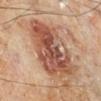Clinical impression:
Recorded during total-body skin imaging; not selected for excision or biopsy.
Acquisition and patient details:
A male subject, in their 70s. Located on the right lower leg. Cropped from a total-body skin-imaging series; the visible field is about 15 mm. Measured at roughly 8 mm in maximum diameter. Automated image analysis of the tile measured a lesion area of about 30 mm², an eccentricity of roughly 0.75, and a shape-asymmetry score of about 0.3 (0 = symmetric). The analysis additionally found an average lesion color of about L≈50 a*≈23 b*≈29 (CIELAB), about 13 CIELAB-L* units darker than the surrounding skin, and a normalized lesion–skin contrast near 9. The software also gave a border-irregularity index near 4.5/10, internal color variation of about 7.5 on a 0–10 scale, and radial color variation of about 2.5. And it measured a nevus-likeness score of about 0/100 and a detector confidence of about 100 out of 100 that the crop contains a lesion.